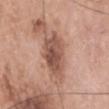The lesion was photographed on a routine skin check and not biopsied; there is no pathology result.
The lesion is located on the head or neck.
A lesion tile, about 15 mm wide, cut from a 3D total-body photograph.
The subject is a male roughly 65 years of age.